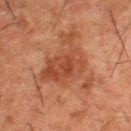The lesion was photographed on a routine skin check and not biopsied; there is no pathology result. Imaged with cross-polarized lighting. Longest diameter approximately 8.5 mm. A male subject, aged 48 to 52. A lesion tile, about 15 mm wide, cut from a 3D total-body photograph. The lesion is located on the upper back.